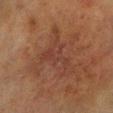Assessment:
No biopsy was performed on this lesion — it was imaged during a full skin examination and was not determined to be concerning.
Background:
The lesion is located on the leg. A 15 mm close-up extracted from a 3D total-body photography capture. A male patient aged approximately 70.